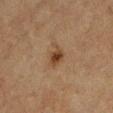The lesion was photographed on a routine skin check and not biopsied; there is no pathology result. An algorithmic analysis of the crop reported an area of roughly 4.5 mm² and a symmetry-axis asymmetry near 0.3. The analysis additionally found a border-irregularity rating of about 3/10 and a within-lesion color-variation index near 3.5/10. It also reported a nevus-likeness score of about 90/100 and a detector confidence of about 100 out of 100 that the crop contains a lesion. Located on the left lower leg. A male subject, aged around 85 or older. The tile uses cross-polarized illumination. A 15 mm close-up tile from a total-body photography series done for melanoma screening. About 2.5 mm across.